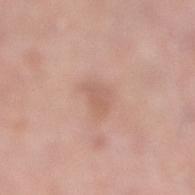Assessment:
This lesion was catalogued during total-body skin photography and was not selected for biopsy.
Background:
The tile uses white-light illumination. A lesion tile, about 15 mm wide, cut from a 3D total-body photograph. A female patient, approximately 55 years of age. Longest diameter approximately 2.5 mm. Located on the leg. The lesion-visualizer software estimated an average lesion color of about L≈60 a*≈21 b*≈27 (CIELAB) and a lesion–skin lightness drop of about 7.